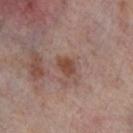Clinical impression: No biopsy was performed on this lesion — it was imaged during a full skin examination and was not determined to be concerning. Image and clinical context: The lesion is on the left thigh. Imaged with cross-polarized lighting. A lesion tile, about 15 mm wide, cut from a 3D total-body photograph. A female patient, in their mid-50s.